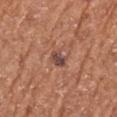Imaged with white-light lighting. A 15 mm close-up tile from a total-body photography series done for melanoma screening. The patient is a female aged 73 to 77. The recorded lesion diameter is about 2.5 mm. Located on the left upper arm. Automated image analysis of the tile measured a lesion area of about 4 mm² and an eccentricity of roughly 0.65. It also reported a mean CIELAB color near L≈46 a*≈21 b*≈24 and a lesion-to-skin contrast of about 9.5 (normalized; higher = more distinct).